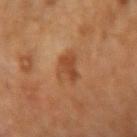Impression:
Imaged during a routine full-body skin examination; the lesion was not biopsied and no histopathology is available.
Clinical summary:
From the right forearm. Longest diameter approximately 3.5 mm. A 15 mm close-up extracted from a 3D total-body photography capture. A female patient, aged 68–72. This is a cross-polarized tile.